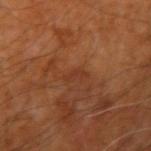<case>
  <patient>
    <sex>male</sex>
    <age_approx>60</age_approx>
  </patient>
  <automated_metrics>
    <area_mm2_approx>2.5</area_mm2_approx>
    <eccentricity>0.95</eccentricity>
    <cielab_L>34</cielab_L>
    <cielab_a>24</cielab_a>
    <cielab_b>31</cielab_b>
    <vs_skin_darker_L>5.0</vs_skin_darker_L>
    <border_irregularity_0_10>5.5</border_irregularity_0_10>
    <color_variation_0_10>0.0</color_variation_0_10>
    <peripheral_color_asymmetry>0.0</peripheral_color_asymmetry>
    <nevus_likeness_0_100>0</nevus_likeness_0_100>
  </automated_metrics>
  <lighting>cross-polarized</lighting>
  <site>arm</site>
  <lesion_size>
    <long_diameter_mm_approx>3.0</long_diameter_mm_approx>
  </lesion_size>
  <image>
    <source>total-body photography crop</source>
    <field_of_view_mm>15</field_of_view_mm>
  </image>
</case>